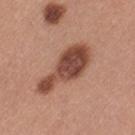Assessment: The lesion was photographed on a routine skin check and not biopsied; there is no pathology result. Context: A roughly 15 mm field-of-view crop from a total-body skin photograph. The lesion is located on the left thigh. The patient is a female approximately 35 years of age.